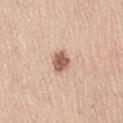Assessment: Recorded during total-body skin imaging; not selected for excision or biopsy. Image and clinical context: Located on the back. A 15 mm close-up tile from a total-body photography series done for melanoma screening. The tile uses white-light illumination. A male patient, approximately 65 years of age.